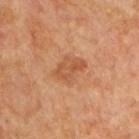follow-up — no biopsy performed (imaged during a skin exam) | site — the chest | tile lighting — cross-polarized | diameter — about 4 mm | subject — male, in their mid- to late 60s | imaging modality — total-body-photography crop, ~15 mm field of view | automated metrics — a shape-asymmetry score of about 0.2 (0 = symmetric); roughly 7 lightness units darker than nearby skin and a lesion-to-skin contrast of about 5.5 (normalized; higher = more distinct); border irregularity of about 2.5 on a 0–10 scale, a within-lesion color-variation index near 3.5/10, and peripheral color asymmetry of about 1; an automated nevus-likeness rating near 0 out of 100 and a detector confidence of about 100 out of 100 that the crop contains a lesion.